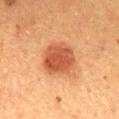{"biopsy_status": "not biopsied; imaged during a skin examination", "site": "mid back", "automated_metrics": {"cielab_L": 50, "cielab_a": 28, "cielab_b": 36, "vs_skin_darker_L": 12.0, "nevus_likeness_0_100": 100, "lesion_detection_confidence_0_100": 100}, "lesion_size": {"long_diameter_mm_approx": 4.0}, "patient": {"sex": "male", "age_approx": 60}, "lighting": "cross-polarized", "image": {"source": "total-body photography crop", "field_of_view_mm": 15}}